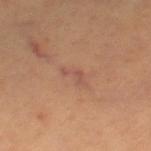Recorded during total-body skin imaging; not selected for excision or biopsy.
The tile uses cross-polarized illumination.
On the right leg.
A lesion tile, about 15 mm wide, cut from a 3D total-body photograph.
About 3 mm across.
A female patient aged 43–47.